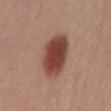The subject is a female in their mid- to late 20s. Located on the mid back. A 15 mm close-up extracted from a 3D total-body photography capture. Measured at roughly 6.5 mm in maximum diameter. On excision, pathology confirmed a Spitz nevus.